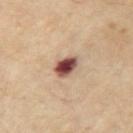No biopsy was performed on this lesion — it was imaged during a full skin examination and was not determined to be concerning. This is a cross-polarized tile. Automated tile analysis of the lesion measured an outline eccentricity of about 0.7 (0 = round, 1 = elongated). The lesion is on the right upper arm. A close-up tile cropped from a whole-body skin photograph, about 15 mm across. The patient is a male aged 68 to 72.